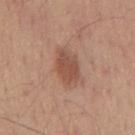| field | value |
|---|---|
| biopsy status | imaged on a skin check; not biopsied |
| imaging modality | ~15 mm tile from a whole-body skin photo |
| patient | male, aged 53 to 57 |
| tile lighting | white-light |
| location | the mid back |
| size | ~4 mm (longest diameter) |